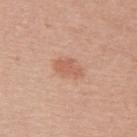Recorded during total-body skin imaging; not selected for excision or biopsy. About 3.5 mm across. Located on the upper back. The tile uses white-light illumination. A female subject, about 50 years old. Automated image analysis of the tile measured border irregularity of about 3 on a 0–10 scale, internal color variation of about 2 on a 0–10 scale, and radial color variation of about 0.5. The analysis additionally found a classifier nevus-likeness of about 70/100. This image is a 15 mm lesion crop taken from a total-body photograph.